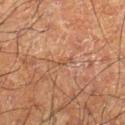Q: Was a biopsy performed?
A: total-body-photography surveillance lesion; no biopsy
Q: Patient demographics?
A: male, aged 58–62
Q: What kind of image is this?
A: ~15 mm crop, total-body skin-cancer survey
Q: Where on the body is the lesion?
A: the arm